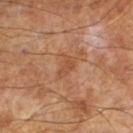The lesion was tiled from a total-body skin photograph and was not biopsied. Cropped from a total-body skin-imaging series; the visible field is about 15 mm. A male subject, roughly 65 years of age. Measured at roughly 3 mm in maximum diameter. Imaged with cross-polarized lighting.